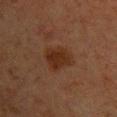workup = imaged on a skin check; not biopsied | image source = total-body-photography crop, ~15 mm field of view | location = the back | subject = female, roughly 55 years of age | illumination = cross-polarized.